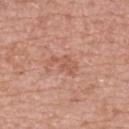Notes:
– follow-up: no biopsy performed (imaged during a skin exam)
– location: the upper back
– lesion diameter: about 4 mm
– lighting: white-light illumination
– patient: male, aged approximately 75
– imaging modality: ~15 mm crop, total-body skin-cancer survey